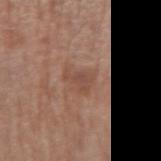Assessment:
Part of a total-body skin-imaging series; this lesion was reviewed on a skin check and was not flagged for biopsy.
Background:
Located on the left forearm. A roughly 15 mm field-of-view crop from a total-body skin photograph. A female subject, in their mid-70s. The tile uses white-light illumination. Automated tile analysis of the lesion measured a lesion color around L≈47 a*≈20 b*≈27 in CIELAB, a lesion–skin lightness drop of about 7, and a normalized lesion–skin contrast near 5.5. The analysis additionally found internal color variation of about 1 on a 0–10 scale and radial color variation of about 0.5. About 3 mm across.